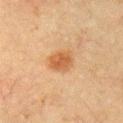Impression: Recorded during total-body skin imaging; not selected for excision or biopsy. Context: The lesion is on the left upper arm. The subject is a male roughly 65 years of age. Longest diameter approximately 3 mm. Imaged with cross-polarized lighting. This image is a 15 mm lesion crop taken from a total-body photograph. The lesion-visualizer software estimated a lesion color around L≈47 a*≈20 b*≈34 in CIELAB and a normalized border contrast of about 7.5. The analysis additionally found internal color variation of about 2.5 on a 0–10 scale.